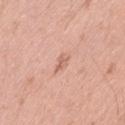tile lighting: white-light illumination
image source: 15 mm crop, total-body photography
patient: female, about 40 years old
site: the lower back